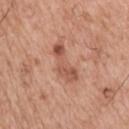The tile uses white-light illumination. A 15 mm crop from a total-body photograph taken for skin-cancer surveillance. The subject is a male aged around 75. Measured at roughly 5 mm in maximum diameter. On the upper back.